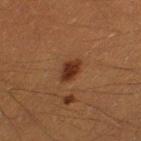  image:
    source: total-body photography crop
    field_of_view_mm: 15
  lesion_size:
    long_diameter_mm_approx: 3.0
  site: left thigh
  patient:
    sex: male
    age_approx: 40
  automated_metrics:
    area_mm2_approx: 5.0
    eccentricity: 0.75
    shape_asymmetry: 0.2
    cielab_L: 26
    cielab_a: 19
    cielab_b: 26
    vs_skin_darker_L: 10.0
    vs_skin_contrast_norm: 10.0
    border_irregularity_0_10: 2.0
    color_variation_0_10: 2.5
    peripheral_color_asymmetry: 0.5